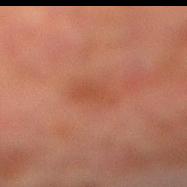notes = catalogued during a skin exam; not biopsied
image source = ~15 mm tile from a whole-body skin photo
lesion size = about 3.5 mm
lighting = cross-polarized illumination
subject = male, aged 73 to 77
anatomic site = the right lower leg